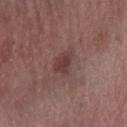| key | value |
|---|---|
| biopsy status | imaged on a skin check; not biopsied |
| TBP lesion metrics | an area of roughly 4.5 mm², an outline eccentricity of about 0.65 (0 = round, 1 = elongated), and a symmetry-axis asymmetry near 0.25; a mean CIELAB color near L≈38 a*≈20 b*≈20, a lesion–skin lightness drop of about 8, and a normalized border contrast of about 7 |
| image source | 15 mm crop, total-body photography |
| lesion diameter | ~2.5 mm (longest diameter) |
| subject | female, aged around 75 |
| location | the right forearm |